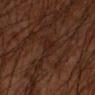Assessment: Part of a total-body skin-imaging series; this lesion was reviewed on a skin check and was not flagged for biopsy. Acquisition and patient details: A roughly 15 mm field-of-view crop from a total-body skin photograph. From the left forearm. The recorded lesion diameter is about 3 mm. The patient is a male aged 58–62. The tile uses cross-polarized illumination.